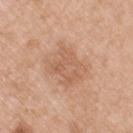The lesion was tiled from a total-body skin photograph and was not biopsied. The lesion-visualizer software estimated a border-irregularity index near 3.5/10, a within-lesion color-variation index near 3/10, and peripheral color asymmetry of about 1. The tile uses white-light illumination. A male patient in their 50s. This image is a 15 mm lesion crop taken from a total-body photograph. The lesion's longest dimension is about 5 mm. On the left upper arm.